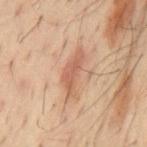Longest diameter approximately 5.5 mm. Captured under cross-polarized illumination. The lesion is on the mid back. A lesion tile, about 15 mm wide, cut from a 3D total-body photograph. The subject is a male aged 58 to 62. An algorithmic analysis of the crop reported an area of roughly 7.5 mm², an eccentricity of roughly 0.95, and a shape-asymmetry score of about 0.45 (0 = symmetric). The software also gave a nevus-likeness score of about 0/100 and a lesion-detection confidence of about 100/100.